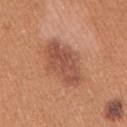{"biopsy_status": "not biopsied; imaged during a skin examination", "lighting": "white-light", "automated_metrics": {"cielab_L": 52, "cielab_a": 25, "cielab_b": 31, "vs_skin_darker_L": 10.0, "vs_skin_contrast_norm": 7.0, "nevus_likeness_0_100": 10}, "patient": {"sex": "female", "age_approx": 40}, "lesion_size": {"long_diameter_mm_approx": 6.0}, "site": "right upper arm", "image": {"source": "total-body photography crop", "field_of_view_mm": 15}}